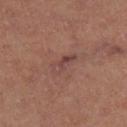Imaged during a routine full-body skin examination; the lesion was not biopsied and no histopathology is available.
A 15 mm crop from a total-body photograph taken for skin-cancer surveillance.
Captured under cross-polarized illumination.
A male subject, in their 60s.
On the right thigh.